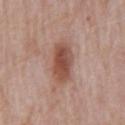Part of a total-body skin-imaging series; this lesion was reviewed on a skin check and was not flagged for biopsy.
Cropped from a whole-body photographic skin survey; the tile spans about 15 mm.
A male subject, in their mid-70s.
Captured under white-light illumination.
On the mid back.
Approximately 4.5 mm at its widest.
An algorithmic analysis of the crop reported an automated nevus-likeness rating near 95 out of 100 and lesion-presence confidence of about 100/100.